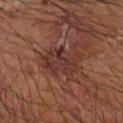| field | value |
|---|---|
| notes | imaged on a skin check; not biopsied |
| image source | ~15 mm crop, total-body skin-cancer survey |
| illumination | cross-polarized |
| lesion diameter | about 5 mm |
| patient | male, aged around 65 |
| body site | the right forearm |